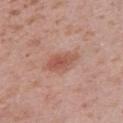The lesion was tiled from a total-body skin photograph and was not biopsied.
About 4 mm across.
The lesion is on the right upper arm.
This image is a 15 mm lesion crop taken from a total-body photograph.
The patient is a male aged 38–42.